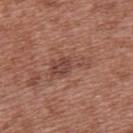Q: Was a biopsy performed?
A: catalogued during a skin exam; not biopsied
Q: Patient demographics?
A: female, aged approximately 40
Q: Illumination type?
A: white-light illumination
Q: What is the imaging modality?
A: ~15 mm tile from a whole-body skin photo
Q: Automated lesion metrics?
A: a mean CIELAB color near L≈45 a*≈23 b*≈25, roughly 8 lightness units darker than nearby skin, and a normalized border contrast of about 6.5; border irregularity of about 6 on a 0–10 scale, internal color variation of about 2.5 on a 0–10 scale, and radial color variation of about 1
Q: Lesion location?
A: the upper back